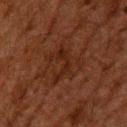| key | value |
|---|---|
| follow-up | catalogued during a skin exam; not biopsied |
| body site | the upper back |
| TBP lesion metrics | a footprint of about 3.5 mm² and two-axis asymmetry of about 0.8; an average lesion color of about L≈18 a*≈19 b*≈22 (CIELAB), about 5 CIELAB-L* units darker than the surrounding skin, and a normalized lesion–skin contrast near 6.5; a within-lesion color-variation index near 0/10 and peripheral color asymmetry of about 0 |
| image source | ~15 mm crop, total-body skin-cancer survey |
| tile lighting | cross-polarized illumination |
| subject | male, in their 60s |
| lesion diameter | about 4 mm |